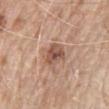– follow-up — imaged on a skin check; not biopsied
– image source — ~15 mm tile from a whole-body skin photo
– patient — male, approximately 80 years of age
– tile lighting — white-light illumination
– site — the mid back